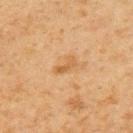| feature | finding |
|---|---|
| biopsy status | no biopsy performed (imaged during a skin exam) |
| image source | ~15 mm tile from a whole-body skin photo |
| site | the left upper arm |
| size | about 2.5 mm |
| patient | male, about 60 years old |
| lighting | cross-polarized |